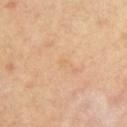The lesion was tiled from a total-body skin photograph and was not biopsied.
The lesion is located on the right upper arm.
This is a cross-polarized tile.
A male subject aged approximately 55.
A close-up tile cropped from a whole-body skin photograph, about 15 mm across.
Approximately 1.5 mm at its widest.
Automated tile analysis of the lesion measured an area of roughly 1.5 mm², an eccentricity of roughly 0.5, and a shape-asymmetry score of about 0.4 (0 = symmetric). It also reported roughly 3 lightness units darker than nearby skin and a lesion-to-skin contrast of about 3 (normalized; higher = more distinct). And it measured a border-irregularity index near 3/10 and radial color variation of about 0. The software also gave a nevus-likeness score of about 0/100 and lesion-presence confidence of about 100/100.